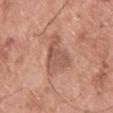<lesion>
<biopsy_status>not biopsied; imaged during a skin examination</biopsy_status>
<site>back</site>
<lighting>white-light</lighting>
<lesion_size>
  <long_diameter_mm_approx>5.0</long_diameter_mm_approx>
</lesion_size>
<patient>
  <sex>male</sex>
  <age_approx>65</age_approx>
</patient>
<image>
  <source>total-body photography crop</source>
  <field_of_view_mm>15</field_of_view_mm>
</image>
<automated_metrics>
  <lesion_detection_confidence_0_100>100</lesion_detection_confidence_0_100>
</automated_metrics>
</lesion>